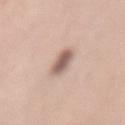This lesion was catalogued during total-body skin photography and was not selected for biopsy.